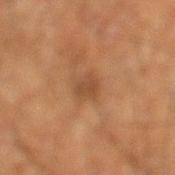  biopsy_status: not biopsied; imaged during a skin examination
  site: leg
  lesion_size:
    long_diameter_mm_approx: 2.5
  automated_metrics:
    area_mm2_approx: 4.0
    eccentricity: 0.7
    shape_asymmetry: 0.3
    cielab_L: 38
    cielab_a: 19
    cielab_b: 29
    vs_skin_darker_L: 7.0
    vs_skin_contrast_norm: 6.0
  image:
    source: total-body photography crop
    field_of_view_mm: 15
  patient:
    sex: male
    age_approx: 65